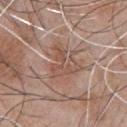biopsy_status: not biopsied; imaged during a skin examination
image:
  source: total-body photography crop
  field_of_view_mm: 15
patient:
  sex: male
  age_approx: 45
lesion_size:
  long_diameter_mm_approx: 4.5
site: front of the torso